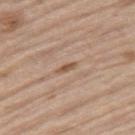biopsy status: imaged on a skin check; not biopsied
automated metrics: a mean CIELAB color near L≈54 a*≈18 b*≈31, roughly 10 lightness units darker than nearby skin, and a normalized lesion–skin contrast near 8; a classifier nevus-likeness of about 0/100 and lesion-presence confidence of about 95/100
location: the right thigh
patient: male, roughly 70 years of age
image source: total-body-photography crop, ~15 mm field of view
diameter: ≈2.5 mm